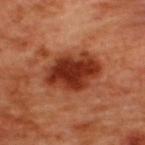Impression: No biopsy was performed on this lesion — it was imaged during a full skin examination and was not determined to be concerning. Clinical summary: Automated image analysis of the tile measured a lesion area of about 20 mm² and two-axis asymmetry of about 0.15. The software also gave an average lesion color of about L≈36 a*≈31 b*≈35 (CIELAB) and a normalized border contrast of about 11.5. The analysis additionally found border irregularity of about 2 on a 0–10 scale, a within-lesion color-variation index near 5.5/10, and radial color variation of about 2. And it measured an automated nevus-likeness rating near 95 out of 100 and a lesion-detection confidence of about 100/100. Cropped from a whole-body photographic skin survey; the tile spans about 15 mm. Captured under cross-polarized illumination. A male subject, aged approximately 50. From the back. The lesion's longest dimension is about 6.5 mm.